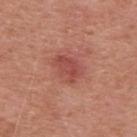Q: Is there a histopathology result?
A: catalogued during a skin exam; not biopsied
Q: How was this image acquired?
A: ~15 mm tile from a whole-body skin photo
Q: What are the patient's age and sex?
A: male, aged around 50
Q: What is the anatomic site?
A: the upper back